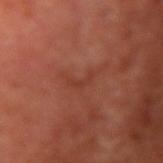workup — total-body-photography surveillance lesion; no biopsy
tile lighting — cross-polarized illumination
acquisition — ~15 mm crop, total-body skin-cancer survey
location — the right upper arm
image-analysis metrics — a lesion area of about 2.5 mm², an eccentricity of roughly 0.9, and a symmetry-axis asymmetry near 0.65; a lesion-to-skin contrast of about 4.5 (normalized; higher = more distinct); a border-irregularity rating of about 7.5/10
size — ≈3 mm
patient — male, aged 63 to 67